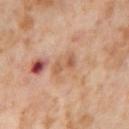Assessment:
Imaged during a routine full-body skin examination; the lesion was not biopsied and no histopathology is available.
Image and clinical context:
Imaged with cross-polarized lighting. A female patient, roughly 55 years of age. On the leg. A lesion tile, about 15 mm wide, cut from a 3D total-body photograph.